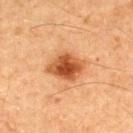Clinical impression:
No biopsy was performed on this lesion — it was imaged during a full skin examination and was not determined to be concerning.
Context:
The patient is a male aged 58 to 62. This is a cross-polarized tile. Longest diameter approximately 4 mm. A 15 mm close-up extracted from a 3D total-body photography capture.